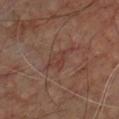<record>
  <biopsy_status>not biopsied; imaged during a skin examination</biopsy_status>
  <site>chest</site>
  <patient>
    <sex>male</sex>
    <age_approx>70</age_approx>
  </patient>
  <image>
    <source>total-body photography crop</source>
    <field_of_view_mm>15</field_of_view_mm>
  </image>
  <lighting>cross-polarized</lighting>
</record>